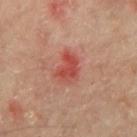This lesion was catalogued during total-body skin photography and was not selected for biopsy.
A roughly 15 mm field-of-view crop from a total-body skin photograph.
The lesion is on the mid back.
A male patient, aged around 65.
The lesion's longest dimension is about 3.5 mm.